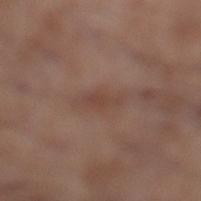biopsy status: catalogued during a skin exam; not biopsied | lighting: white-light | anatomic site: the left lower leg | image: ~15 mm tile from a whole-body skin photo | diameter: about 3 mm | patient: male, in their mid- to late 50s | automated metrics: an average lesion color of about L≈43 a*≈19 b*≈25 (CIELAB), roughly 6 lightness units darker than nearby skin, and a normalized border contrast of about 5.5; a border-irregularity rating of about 4.5/10, a within-lesion color-variation index near 0.5/10, and a peripheral color-asymmetry measure near 0.